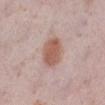Q: What is the lesion's diameter?
A: ≈4 mm
Q: What kind of image is this?
A: ~15 mm tile from a whole-body skin photo
Q: What did automated image analysis measure?
A: an area of roughly 9.5 mm², a shape eccentricity near 0.65, and a symmetry-axis asymmetry near 0.15; a border-irregularity rating of about 1/10, internal color variation of about 2.5 on a 0–10 scale, and radial color variation of about 1
Q: Illumination type?
A: white-light illumination
Q: What are the patient's age and sex?
A: female, aged approximately 40
Q: What is the anatomic site?
A: the right lower leg